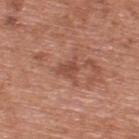Clinical impression:
Recorded during total-body skin imaging; not selected for excision or biopsy.
Acquisition and patient details:
The lesion is on the upper back. A male patient, roughly 70 years of age. Approximately 3.5 mm at its widest. Captured under white-light illumination. A close-up tile cropped from a whole-body skin photograph, about 15 mm across.